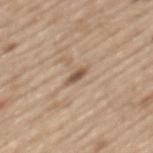Imaged with white-light lighting.
The lesion is on the mid back.
A male patient aged 68 to 72.
A roughly 15 mm field-of-view crop from a total-body skin photograph.